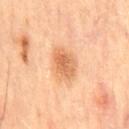- follow-up: catalogued during a skin exam; not biopsied
- illumination: cross-polarized illumination
- lesion diameter: ~4 mm (longest diameter)
- imaging modality: ~15 mm crop, total-body skin-cancer survey
- patient: male, approximately 65 years of age
- location: the chest
- image-analysis metrics: a lesion area of about 10 mm², a shape eccentricity near 0.65, and a shape-asymmetry score of about 0.2 (0 = symmetric); a mean CIELAB color near L≈67 a*≈24 b*≈40, about 11 CIELAB-L* units darker than the surrounding skin, and a normalized border contrast of about 7